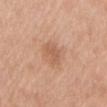Imaged during a routine full-body skin examination; the lesion was not biopsied and no histopathology is available.
A male subject, aged around 50.
Approximately 2.5 mm at its widest.
The lesion is on the chest.
The total-body-photography lesion software estimated an area of roughly 3.5 mm² and an outline eccentricity of about 0.65 (0 = round, 1 = elongated). And it measured a classifier nevus-likeness of about 5/100 and lesion-presence confidence of about 100/100.
A 15 mm close-up tile from a total-body photography series done for melanoma screening.